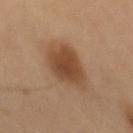The lesion was tiled from a total-body skin photograph and was not biopsied. Cropped from a whole-body photographic skin survey; the tile spans about 15 mm. A male subject, roughly 60 years of age. On the mid back. An algorithmic analysis of the crop reported an area of roughly 16 mm², a shape eccentricity near 0.8, and two-axis asymmetry of about 0.2. Measured at roughly 6 mm in maximum diameter. Captured under cross-polarized illumination.